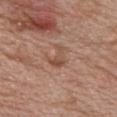This lesion was catalogued during total-body skin photography and was not selected for biopsy. Automated image analysis of the tile measured a shape eccentricity near 0.8. The analysis additionally found a mean CIELAB color near L≈50 a*≈21 b*≈29, roughly 8 lightness units darker than nearby skin, and a normalized border contrast of about 6. The software also gave border irregularity of about 6.5 on a 0–10 scale and radial color variation of about 0.5. Imaged with white-light lighting. A male subject aged 68–72. A close-up tile cropped from a whole-body skin photograph, about 15 mm across. The lesion is on the front of the torso.